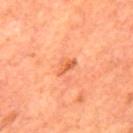{"biopsy_status": "not biopsied; imaged during a skin examination", "lesion_size": {"long_diameter_mm_approx": 3.0}, "lighting": "cross-polarized", "image": {"source": "total-body photography crop", "field_of_view_mm": 15}, "patient": {"sex": "male", "age_approx": 65}, "site": "mid back"}